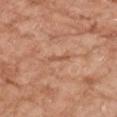Part of a total-body skin-imaging series; this lesion was reviewed on a skin check and was not flagged for biopsy. The subject is a female aged around 75. From the right forearm. This is a white-light tile. Cropped from a whole-body photographic skin survey; the tile spans about 15 mm.